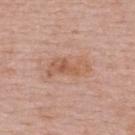A region of skin cropped from a whole-body photographic capture, roughly 15 mm wide. On the upper back. The recorded lesion diameter is about 5 mm. Automated image analysis of the tile measured a border-irregularity rating of about 5.5/10 and peripheral color asymmetry of about 1. And it measured an automated nevus-likeness rating near 0 out of 100 and a lesion-detection confidence of about 100/100. A female patient aged approximately 50.